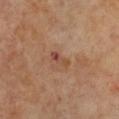Clinical impression: Imaged during a routine full-body skin examination; the lesion was not biopsied and no histopathology is available. Clinical summary: About 2.5 mm across. A female subject, in their mid-60s. Located on the chest. The total-body-photography lesion software estimated a footprint of about 2.5 mm² and an outline eccentricity of about 0.9 (0 = round, 1 = elongated). It also reported an average lesion color of about L≈45 a*≈22 b*≈29 (CIELAB), about 8 CIELAB-L* units darker than the surrounding skin, and a normalized lesion–skin contrast near 6.5. The analysis additionally found a border-irregularity rating of about 4.5/10 and a color-variation rating of about 0/10. Cropped from a total-body skin-imaging series; the visible field is about 15 mm.